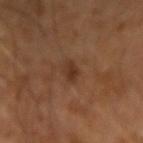Impression:
Imaged during a routine full-body skin examination; the lesion was not biopsied and no histopathology is available.